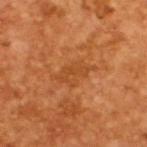A region of skin cropped from a whole-body photographic capture, roughly 15 mm wide. The patient is a male roughly 65 years of age.